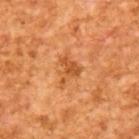Assessment:
No biopsy was performed on this lesion — it was imaged during a full skin examination and was not determined to be concerning.
Context:
The total-body-photography lesion software estimated a symmetry-axis asymmetry near 0.6. It also reported a lesion color around L≈53 a*≈29 b*≈45 in CIELAB, a lesion–skin lightness drop of about 10, and a normalized border contrast of about 7. The analysis additionally found border irregularity of about 6.5 on a 0–10 scale, a within-lesion color-variation index near 1.5/10, and radial color variation of about 0.5. A male subject, in their mid- to late 60s. A roughly 15 mm field-of-view crop from a total-body skin photograph. Captured under cross-polarized illumination. The recorded lesion diameter is about 3.5 mm.